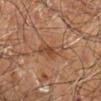The lesion was tiled from a total-body skin photograph and was not biopsied. The subject is a male aged around 70. Longest diameter approximately 5 mm. Imaged with cross-polarized lighting. The lesion is on the left forearm. A 15 mm crop from a total-body photograph taken for skin-cancer surveillance.